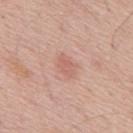{"patient": {"sex": "male", "age_approx": 55}, "lighting": "white-light", "site": "back", "lesion_size": {"long_diameter_mm_approx": 3.0}, "automated_metrics": {"cielab_L": 61, "cielab_a": 23, "cielab_b": 27, "vs_skin_contrast_norm": 5.0, "lesion_detection_confidence_0_100": 100}, "image": {"source": "total-body photography crop", "field_of_view_mm": 15}}